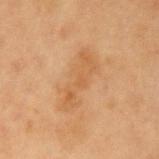automated lesion analysis — a shape eccentricity near 0.95 and a shape-asymmetry score of about 0.3 (0 = symmetric); a detector confidence of about 100 out of 100 that the crop contains a lesion | patient — female, aged around 40 | lighting — cross-polarized illumination | image — ~15 mm tile from a whole-body skin photo | location — the left upper arm.